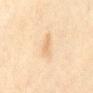biopsy status: imaged on a skin check; not biopsied
tile lighting: cross-polarized
site: the lower back
subject: female, aged approximately 40
automated lesion analysis: a footprint of about 3.5 mm² and a shape eccentricity near 0.85; a border-irregularity rating of about 3.5/10, internal color variation of about 1.5 on a 0–10 scale, and radial color variation of about 0.5; a nevus-likeness score of about 0/100 and lesion-presence confidence of about 100/100
image: ~15 mm tile from a whole-body skin photo
lesion size: ≈3 mm